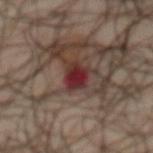follow-up=total-body-photography surveillance lesion; no biopsy | tile lighting=cross-polarized | imaging modality=~15 mm tile from a whole-body skin photo | lesion size=≈3.5 mm | patient=male, approximately 65 years of age | location=the abdomen.